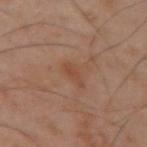biopsy status = total-body-photography surveillance lesion; no biopsy
site = the arm
subject = male, aged 48 to 52
illumination = cross-polarized illumination
acquisition = total-body-photography crop, ~15 mm field of view
size = ≈2.5 mm